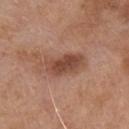Assessment: No biopsy was performed on this lesion — it was imaged during a full skin examination and was not determined to be concerning. Clinical summary: A male subject roughly 80 years of age. A 15 mm close-up tile from a total-body photography series done for melanoma screening. Approximately 5 mm at its widest. The total-body-photography lesion software estimated a lesion color around L≈47 a*≈22 b*≈29 in CIELAB, roughly 11 lightness units darker than nearby skin, and a normalized lesion–skin contrast near 8. The tile uses white-light illumination. The lesion is located on the chest.